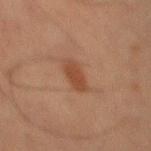Recorded during total-body skin imaging; not selected for excision or biopsy.
A 15 mm crop from a total-body photograph taken for skin-cancer surveillance.
Measured at roughly 4 mm in maximum diameter.
Located on the abdomen.
An algorithmic analysis of the crop reported border irregularity of about 2.5 on a 0–10 scale, a color-variation rating of about 1.5/10, and peripheral color asymmetry of about 0.5. The software also gave an automated nevus-likeness rating near 95 out of 100 and a detector confidence of about 100 out of 100 that the crop contains a lesion.
Captured under cross-polarized illumination.
The patient is a male aged around 65.